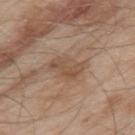The lesion was tiled from a total-body skin photograph and was not biopsied. A male subject approximately 55 years of age. From the left upper arm. Cropped from a whole-body photographic skin survey; the tile spans about 15 mm.